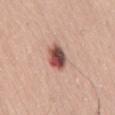follow-up: no biopsy performed (imaged during a skin exam)
illumination: white-light
imaging modality: ~15 mm crop, total-body skin-cancer survey
size: ≈3.5 mm
subject: male, aged around 60
site: the back